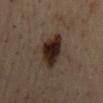notes = imaged on a skin check; not biopsied | acquisition = total-body-photography crop, ~15 mm field of view | illumination = cross-polarized illumination | anatomic site = the mid back | lesion diameter = ≈6 mm | subject = male, aged 53–57.